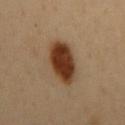follow-up = imaged on a skin check; not biopsied | patient = male, about 50 years old | lighting = cross-polarized illumination | image source = ~15 mm crop, total-body skin-cancer survey | lesion diameter = ≈6 mm | site = the mid back.